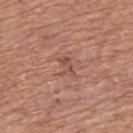The total-body-photography lesion software estimated a lesion color around L≈51 a*≈23 b*≈28 in CIELAB and a lesion–skin lightness drop of about 7. The software also gave a border-irregularity index near 5.5/10, internal color variation of about 2 on a 0–10 scale, and a peripheral color-asymmetry measure near 0.5. A male patient, approximately 65 years of age. Approximately 2.5 mm at its widest. The lesion is on the upper back. A lesion tile, about 15 mm wide, cut from a 3D total-body photograph. Imaged with white-light lighting.